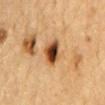This lesion was catalogued during total-body skin photography and was not selected for biopsy. Automated tile analysis of the lesion measured an average lesion color of about L≈40 a*≈21 b*≈33 (CIELAB), roughly 18 lightness units darker than nearby skin, and a normalized border contrast of about 14. A region of skin cropped from a whole-body photographic capture, roughly 15 mm wide. The patient is a male aged 83 or older. Imaged with cross-polarized lighting. Located on the mid back. The lesion's longest dimension is about 3 mm.